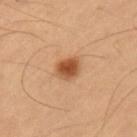automated lesion analysis = a shape eccentricity near 0.5 and two-axis asymmetry of about 0.25; border irregularity of about 2 on a 0–10 scale, a color-variation rating of about 3.5/10, and peripheral color asymmetry of about 1
image = ~15 mm tile from a whole-body skin photo
patient = male, roughly 55 years of age
lesion diameter = about 3 mm
lighting = cross-polarized illumination
body site = the left upper arm